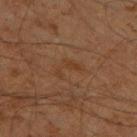Q: What are the patient's age and sex?
A: male, in their 60s
Q: How was this image acquired?
A: ~15 mm crop, total-body skin-cancer survey
Q: Where on the body is the lesion?
A: the left thigh